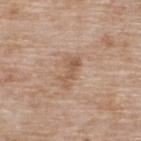biopsy status: no biopsy performed (imaged during a skin exam) | automated lesion analysis: a lesion color around L≈56 a*≈18 b*≈31 in CIELAB, about 8 CIELAB-L* units darker than the surrounding skin, and a normalized lesion–skin contrast near 6 | imaging modality: 15 mm crop, total-body photography | subject: female, about 75 years old | size: ≈4 mm | site: the upper back.